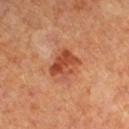Assessment:
This lesion was catalogued during total-body skin photography and was not selected for biopsy.
Clinical summary:
This is a cross-polarized tile. Located on the leg. A female patient. A roughly 15 mm field-of-view crop from a total-body skin photograph. Automated tile analysis of the lesion measured an automated nevus-likeness rating near 85 out of 100 and a lesion-detection confidence of about 100/100. Longest diameter approximately 3.5 mm.